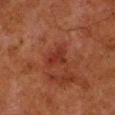follow-up: no biopsy performed (imaged during a skin exam)
location: the left lower leg
patient: male, aged approximately 80
TBP lesion metrics: a mean CIELAB color near L≈26 a*≈24 b*≈25, about 6 CIELAB-L* units darker than the surrounding skin, and a lesion-to-skin contrast of about 6.5 (normalized; higher = more distinct); a border-irregularity index near 5/10, a color-variation rating of about 3/10, and a peripheral color-asymmetry measure near 1
illumination: cross-polarized
acquisition: total-body-photography crop, ~15 mm field of view
diameter: ≈3 mm